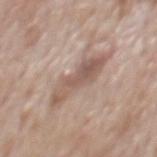| feature | finding |
|---|---|
| biopsy status | imaged on a skin check; not biopsied |
| lesion diameter | ~7 mm (longest diameter) |
| body site | the back |
| subject | male, aged 68 to 72 |
| imaging modality | 15 mm crop, total-body photography |